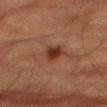biopsy status = catalogued during a skin exam; not biopsied
patient = male, in their mid-80s or older
image source = ~15 mm tile from a whole-body skin photo
image-analysis metrics = a footprint of about 5 mm² and a symmetry-axis asymmetry near 0.2; a nevus-likeness score of about 95/100 and a detector confidence of about 100 out of 100 that the crop contains a lesion
lesion diameter = about 2.5 mm
anatomic site = the right thigh